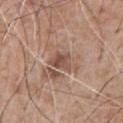• workup: no biopsy performed (imaged during a skin exam)
• acquisition: ~15 mm tile from a whole-body skin photo
• image-analysis metrics: a shape eccentricity near 0.6; a lesion-detection confidence of about 100/100
• patient: male, in their 60s
• illumination: white-light
• site: the chest